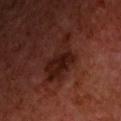Impression:
Imaged during a routine full-body skin examination; the lesion was not biopsied and no histopathology is available.
Image and clinical context:
The patient is a male aged around 60. Cropped from a total-body skin-imaging series; the visible field is about 15 mm. Approximately 6 mm at its widest. From the chest.